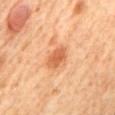Context:
A female subject, aged 63–67. A 15 mm close-up extracted from a 3D total-body photography capture. The lesion is located on the back.